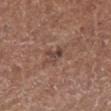<lesion>
  <biopsy_status>not biopsied; imaged during a skin examination</biopsy_status>
  <lesion_size>
    <long_diameter_mm_approx>2.5</long_diameter_mm_approx>
  </lesion_size>
  <image>
    <source>total-body photography crop</source>
    <field_of_view_mm>15</field_of_view_mm>
  </image>
  <lighting>white-light</lighting>
  <site>left lower leg</site>
  <patient>
    <sex>male</sex>
    <age_approx>75</age_approx>
  </patient>
</lesion>